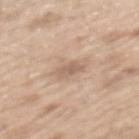workup — total-body-photography surveillance lesion; no biopsy
image — total-body-photography crop, ~15 mm field of view
location — the mid back
patient — male, aged 68–72